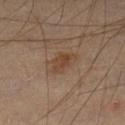Recorded during total-body skin imaging; not selected for excision or biopsy. Automated image analysis of the tile measured an area of roughly 5 mm², an outline eccentricity of about 0.8 (0 = round, 1 = elongated), and a shape-asymmetry score of about 0.4 (0 = symmetric). The analysis additionally found a lesion color around L≈42 a*≈18 b*≈28 in CIELAB and about 7 CIELAB-L* units darker than the surrounding skin. A 15 mm close-up extracted from a 3D total-body photography capture. Measured at roughly 3.5 mm in maximum diameter. The patient is a male aged approximately 45. The tile uses cross-polarized illumination. From the left lower leg.